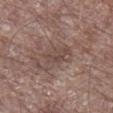Imaged during a routine full-body skin examination; the lesion was not biopsied and no histopathology is available. This is a white-light tile. This image is a 15 mm lesion crop taken from a total-body photograph. An algorithmic analysis of the crop reported a footprint of about 6.5 mm² and a shape eccentricity near 0.8. The analysis additionally found internal color variation of about 2 on a 0–10 scale and a peripheral color-asymmetry measure near 1. The software also gave a nevus-likeness score of about 0/100 and a detector confidence of about 85 out of 100 that the crop contains a lesion. A male subject aged approximately 70. Approximately 4 mm at its widest. Located on the right lower leg.